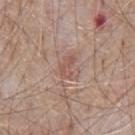| field | value |
|---|---|
| notes | imaged on a skin check; not biopsied |
| patient | male, about 65 years old |
| image-analysis metrics | a footprint of about 4 mm², an eccentricity of roughly 0.8, and two-axis asymmetry of about 0.45; a mean CIELAB color near L≈54 a*≈21 b*≈25, about 7 CIELAB-L* units darker than the surrounding skin, and a normalized border contrast of about 5 |
| image | 15 mm crop, total-body photography |
| diameter | about 2.5 mm |
| lighting | white-light |
| body site | the front of the torso |